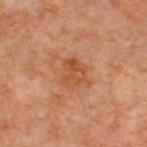Part of a total-body skin-imaging series; this lesion was reviewed on a skin check and was not flagged for biopsy.
A male patient, aged approximately 65.
A close-up tile cropped from a whole-body skin photograph, about 15 mm across.
The lesion is on the chest.